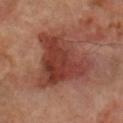An algorithmic analysis of the crop reported a border-irregularity index near 5.5/10, a within-lesion color-variation index near 5.5/10, and radial color variation of about 2. And it measured a classifier nevus-likeness of about 60/100. The recorded lesion diameter is about 7.5 mm. The lesion is located on the left forearm. The patient is a male in their 70s. Cropped from a total-body skin-imaging series; the visible field is about 15 mm.